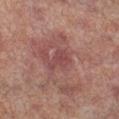Q: Is there a histopathology result?
A: no biopsy performed (imaged during a skin exam)
Q: Illumination type?
A: cross-polarized
Q: How was this image acquired?
A: total-body-photography crop, ~15 mm field of view
Q: What did automated image analysis measure?
A: an area of roughly 4 mm² and a shape eccentricity near 0.75; a classifier nevus-likeness of about 0/100 and a detector confidence of about 100 out of 100 that the crop contains a lesion
Q: Lesion size?
A: about 3 mm
Q: Who is the patient?
A: male, roughly 65 years of age
Q: Lesion location?
A: the right lower leg